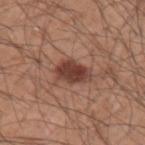Located on the left upper arm. The lesion-visualizer software estimated an area of roughly 8.5 mm², an outline eccentricity of about 0.85 (0 = round, 1 = elongated), and two-axis asymmetry of about 0.15. The analysis additionally found border irregularity of about 2 on a 0–10 scale, internal color variation of about 3.5 on a 0–10 scale, and peripheral color asymmetry of about 1. And it measured a nevus-likeness score of about 95/100 and a lesion-detection confidence of about 100/100. A roughly 15 mm field-of-view crop from a total-body skin photograph. The recorded lesion diameter is about 4 mm. A male subject, aged 58–62. Captured under white-light illumination.